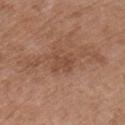follow-up: total-body-photography surveillance lesion; no biopsy
tile lighting: white-light illumination
image source: 15 mm crop, total-body photography
lesion diameter: ~3 mm (longest diameter)
location: the chest
subject: male, roughly 65 years of age
TBP lesion metrics: a lesion color around L≈47 a*≈21 b*≈30 in CIELAB, a lesion–skin lightness drop of about 6, and a normalized border contrast of about 5; a border-irregularity index near 4.5/10, a within-lesion color-variation index near 2.5/10, and radial color variation of about 1; a classifier nevus-likeness of about 0/100